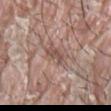{"biopsy_status": "not biopsied; imaged during a skin examination", "patient": {"sex": "male", "age_approx": 40}, "site": "upper back", "image": {"source": "total-body photography crop", "field_of_view_mm": 15}, "lighting": "white-light", "lesion_size": {"long_diameter_mm_approx": 2.5}}